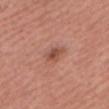Image and clinical context: The lesion's longest dimension is about 3 mm. A 15 mm crop from a total-body photograph taken for skin-cancer surveillance. The lesion is on the chest. Captured under white-light illumination. Automated tile analysis of the lesion measured a lesion color around L≈51 a*≈25 b*≈29 in CIELAB, roughly 10 lightness units darker than nearby skin, and a normalized border contrast of about 7. And it measured radial color variation of about 1. It also reported an automated nevus-likeness rating near 55 out of 100 and lesion-presence confidence of about 100/100. The subject is a male approximately 50 years of age.